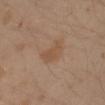Case summary:
• notes: no biopsy performed (imaged during a skin exam)
• body site: the left arm
• imaging modality: ~15 mm crop, total-body skin-cancer survey
• diameter: ≈3.5 mm
• tile lighting: cross-polarized
• patient: male, aged 28 to 32